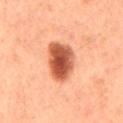Case summary:
• workup: catalogued during a skin exam; not biopsied
• tile lighting: cross-polarized illumination
• subject: male, aged approximately 60
• site: the mid back
• image source: ~15 mm crop, total-body skin-cancer survey